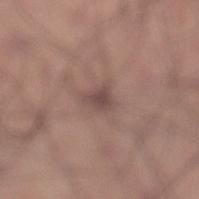The lesion was tiled from a total-body skin photograph and was not biopsied.
Cropped from a total-body skin-imaging series; the visible field is about 15 mm.
The patient is a male aged 23–27.
On the left lower leg.
Captured under white-light illumination.
Measured at roughly 3 mm in maximum diameter.